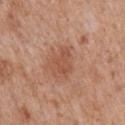Notes:
– biopsy status: imaged on a skin check; not biopsied
– body site: the mid back
– patient: male, approximately 65 years of age
– imaging modality: total-body-photography crop, ~15 mm field of view